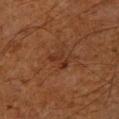Impression: The lesion was tiled from a total-body skin photograph and was not biopsied. Acquisition and patient details: Captured under cross-polarized illumination. The lesion is on the left forearm. Automated tile analysis of the lesion measured an average lesion color of about L≈27 a*≈20 b*≈27 (CIELAB), roughly 5 lightness units darker than nearby skin, and a normalized lesion–skin contrast near 6. A male subject, in their 60s. The lesion's longest dimension is about 2.5 mm. Cropped from a total-body skin-imaging series; the visible field is about 15 mm.